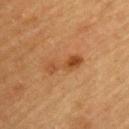- workup — no biopsy performed (imaged during a skin exam)
- imaging modality — total-body-photography crop, ~15 mm field of view
- illumination — cross-polarized illumination
- subject — male, roughly 60 years of age
- size — ~5 mm (longest diameter)
- location — the front of the torso